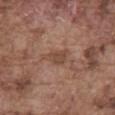The lesion was photographed on a routine skin check and not biopsied; there is no pathology result. A region of skin cropped from a whole-body photographic capture, roughly 15 mm wide. A male subject roughly 75 years of age. The lesion is on the abdomen.